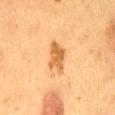{
  "biopsy_status": "not biopsied; imaged during a skin examination",
  "patient": {
    "sex": "female",
    "age_approx": 50
  },
  "site": "lower back",
  "image": {
    "source": "total-body photography crop",
    "field_of_view_mm": 15
  },
  "lighting": "cross-polarized"
}